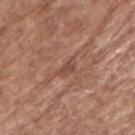Clinical impression: The lesion was tiled from a total-body skin photograph and was not biopsied. Background: The recorded lesion diameter is about 2.5 mm. A lesion tile, about 15 mm wide, cut from a 3D total-body photograph. The subject is a male aged 73–77. Imaged with white-light lighting. Located on the right upper arm. The lesion-visualizer software estimated a lesion color around L≈47 a*≈23 b*≈28 in CIELAB, about 8 CIELAB-L* units darker than the surrounding skin, and a normalized lesion–skin contrast near 6. And it measured a nevus-likeness score of about 0/100 and a detector confidence of about 75 out of 100 that the crop contains a lesion.